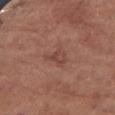Case summary:
• biopsy status — catalogued during a skin exam; not biopsied
• size — ≈3 mm
• image — total-body-photography crop, ~15 mm field of view
• illumination — white-light illumination
• site — the arm
• patient — female, approximately 75 years of age